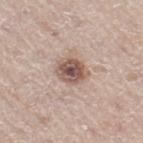Q: Is there a histopathology result?
A: catalogued during a skin exam; not biopsied
Q: Automated lesion metrics?
A: a footprint of about 8 mm², an eccentricity of roughly 0.6, and a symmetry-axis asymmetry near 0.15
Q: Where on the body is the lesion?
A: the right thigh
Q: What is the lesion's diameter?
A: about 3.5 mm
Q: What kind of image is this?
A: 15 mm crop, total-body photography
Q: Who is the patient?
A: male, aged around 65
Q: What lighting was used for the tile?
A: white-light illumination